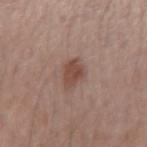Captured during whole-body skin photography for melanoma surveillance; the lesion was not biopsied.
The lesion is located on the left forearm.
Automated image analysis of the tile measured a classifier nevus-likeness of about 70/100 and lesion-presence confidence of about 100/100.
Cropped from a whole-body photographic skin survey; the tile spans about 15 mm.
A female subject aged 53 to 57.